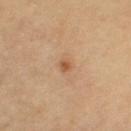Impression: The lesion was tiled from a total-body skin photograph and was not biopsied. Context: A female subject, roughly 65 years of age. A region of skin cropped from a whole-body photographic capture, roughly 15 mm wide. Captured under cross-polarized illumination. Automated tile analysis of the lesion measured an eccentricity of roughly 0.65 and a shape-asymmetry score of about 0.35 (0 = symmetric). And it measured a peripheral color-asymmetry measure near 0. And it measured an automated nevus-likeness rating near 45 out of 100 and a detector confidence of about 100 out of 100 that the crop contains a lesion. From the left upper arm.